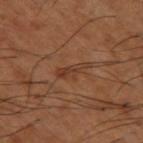  biopsy_status: not biopsied; imaged during a skin examination
  image:
    source: total-body photography crop
    field_of_view_mm: 15
  lesion_size:
    long_diameter_mm_approx: 4.0
  patient:
    sex: male
    age_approx: 65
  automated_metrics:
    area_mm2_approx: 4.5
    eccentricity: 0.9
    shape_asymmetry: 0.45
    vs_skin_contrast_norm: 6.0
    nevus_likeness_0_100: 0
    lesion_detection_confidence_0_100: 95
  site: leg
  lighting: cross-polarized